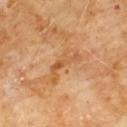<tbp_lesion>
<patient>
  <sex>male</sex>
  <age_approx>60</age_approx>
</patient>
<site>chest</site>
<image>
  <source>total-body photography crop</source>
  <field_of_view_mm>15</field_of_view_mm>
</image>
</tbp_lesion>